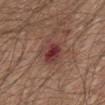Impression: The lesion was photographed on a routine skin check and not biopsied; there is no pathology result. Background: A male subject, aged approximately 65. The lesion is located on the abdomen. The tile uses white-light illumination. Approximately 3.5 mm at its widest. This image is a 15 mm lesion crop taken from a total-body photograph. Automated tile analysis of the lesion measured an area of roughly 7.5 mm², an eccentricity of roughly 0.55, and two-axis asymmetry of about 0.2. The analysis additionally found a border-irregularity rating of about 2/10, internal color variation of about 6.5 on a 0–10 scale, and radial color variation of about 1.5.